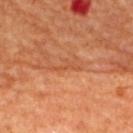No biopsy was performed on this lesion — it was imaged during a full skin examination and was not determined to be concerning. Captured under cross-polarized illumination. Cropped from a whole-body photographic skin survey; the tile spans about 15 mm. Approximately 4 mm at its widest. A female patient aged 63–67. On the upper back.